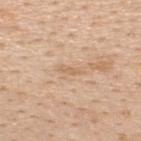{
  "biopsy_status": "not biopsied; imaged during a skin examination",
  "lesion_size": {
    "long_diameter_mm_approx": 2.5
  },
  "lighting": "white-light",
  "automated_metrics": {
    "area_mm2_approx": 2.0,
    "eccentricity": 0.95,
    "shape_asymmetry": 0.25,
    "cielab_L": 64,
    "cielab_a": 18,
    "cielab_b": 35,
    "vs_skin_darker_L": 7.0,
    "vs_skin_contrast_norm": 5.0,
    "border_irregularity_0_10": 3.5,
    "color_variation_0_10": 0.0,
    "peripheral_color_asymmetry": 0.0,
    "nevus_likeness_0_100": 0,
    "lesion_detection_confidence_0_100": 95
  },
  "image": {
    "source": "total-body photography crop",
    "field_of_view_mm": 15
  },
  "patient": {
    "sex": "male",
    "age_approx": 50
  },
  "site": "back"
}